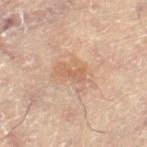| field | value |
|---|---|
| follow-up | imaged on a skin check; not biopsied |
| anatomic site | the right leg |
| imaging modality | ~15 mm crop, total-body skin-cancer survey |
| patient | female, aged 78–82 |